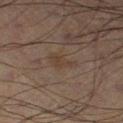Q: Is there a histopathology result?
A: imaged on a skin check; not biopsied
Q: Who is the patient?
A: male, aged around 70
Q: What is the anatomic site?
A: the leg
Q: What did automated image analysis measure?
A: a normalized border contrast of about 5; a color-variation rating of about 1/10 and peripheral color asymmetry of about 0.5
Q: What is the imaging modality?
A: total-body-photography crop, ~15 mm field of view
Q: How was the tile lit?
A: cross-polarized illumination
Q: How large is the lesion?
A: ~3.5 mm (longest diameter)